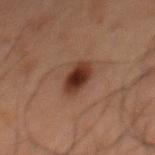Recorded during total-body skin imaging; not selected for excision or biopsy. Located on the mid back. A close-up tile cropped from a whole-body skin photograph, about 15 mm across. The patient is a male in their 60s. Imaged with cross-polarized lighting. The recorded lesion diameter is about 4 mm.